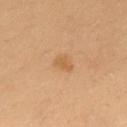follow-up = total-body-photography surveillance lesion; no biopsy
acquisition = 15 mm crop, total-body photography
site = the back
lesion diameter = ≈2.5 mm
subject = female, aged around 40
automated metrics = border irregularity of about 3.5 on a 0–10 scale, a color-variation rating of about 0.5/10, and a peripheral color-asymmetry measure near 0